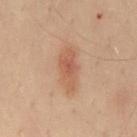| feature | finding |
|---|---|
| follow-up | total-body-photography surveillance lesion; no biopsy |
| image-analysis metrics | a lesion color around L≈46 a*≈18 b*≈27 in CIELAB, a lesion–skin lightness drop of about 7, and a lesion-to-skin contrast of about 6 (normalized; higher = more distinct); border irregularity of about 2.5 on a 0–10 scale, internal color variation of about 3.5 on a 0–10 scale, and peripheral color asymmetry of about 1; lesion-presence confidence of about 100/100 |
| patient | male, aged 48–52 |
| acquisition | ~15 mm tile from a whole-body skin photo |
| lesion diameter | ~5.5 mm (longest diameter) |
| site | the mid back |